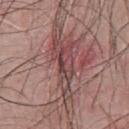Clinical impression:
Imaged during a routine full-body skin examination; the lesion was not biopsied and no histopathology is available.
Background:
From the upper back. The patient is a male in their mid- to late 40s. Imaged with white-light lighting. Cropped from a whole-body photographic skin survey; the tile spans about 15 mm.